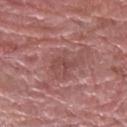No biopsy was performed on this lesion — it was imaged during a full skin examination and was not determined to be concerning.